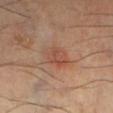Case summary:
– imaging modality: total-body-photography crop, ~15 mm field of view
– tile lighting: cross-polarized
– automated lesion analysis: an eccentricity of roughly 0.65 and a symmetry-axis asymmetry near 0.15
– patient: male, approximately 40 years of age
– anatomic site: the left lower leg
– diameter: ~3.5 mm (longest diameter)